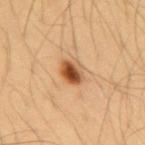follow-up: no biopsy performed (imaged during a skin exam); size: ≈3.5 mm; image source: ~15 mm tile from a whole-body skin photo; subject: male, in their mid- to late 50s; tile lighting: cross-polarized; body site: the back.